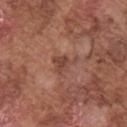Recorded during total-body skin imaging; not selected for excision or biopsy. A 15 mm close-up tile from a total-body photography series done for melanoma screening. Imaged with white-light lighting. Located on the arm. Longest diameter approximately 3 mm. A male subject in their mid-70s.